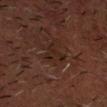The lesion was tiled from a total-body skin photograph and was not biopsied.
This is a cross-polarized tile.
A male patient, aged 58–62.
The lesion is on the head or neck.
A 15 mm crop from a total-body photograph taken for skin-cancer surveillance.